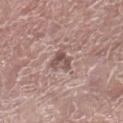Recorded during total-body skin imaging; not selected for excision or biopsy. A male subject aged 73 to 77. The lesion-visualizer software estimated an automated nevus-likeness rating near 0 out of 100. Captured under white-light illumination. Longest diameter approximately 3 mm. This image is a 15 mm lesion crop taken from a total-body photograph. Located on the right lower leg.